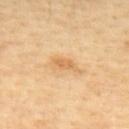biopsy status: no biopsy performed (imaged during a skin exam); anatomic site: the upper back; image source: 15 mm crop, total-body photography; patient: female, aged 43 to 47.